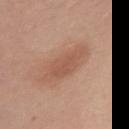No biopsy was performed on this lesion — it was imaged during a full skin examination and was not determined to be concerning. From the right upper arm. The subject is a female about 30 years old. This is a white-light tile. An algorithmic analysis of the crop reported an area of roughly 11 mm², an outline eccentricity of about 0.85 (0 = round, 1 = elongated), and a shape-asymmetry score of about 0.25 (0 = symmetric). The software also gave a border-irregularity index near 3.5/10 and a within-lesion color-variation index near 2/10. And it measured a classifier nevus-likeness of about 80/100 and lesion-presence confidence of about 100/100. This image is a 15 mm lesion crop taken from a total-body photograph. Approximately 5.5 mm at its widest.